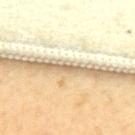<case>
  <biopsy_status>not biopsied; imaged during a skin examination</biopsy_status>
  <image>
    <source>total-body photography crop</source>
    <field_of_view_mm>15</field_of_view_mm>
  </image>
  <patient>
    <sex>female</sex>
    <age_approx>60</age_approx>
  </patient>
  <lighting>cross-polarized</lighting>
  <site>upper back</site>
  <automated_metrics>
    <area_mm2_approx>1.0</area_mm2_approx>
    <shape_asymmetry>0.55</shape_asymmetry>
  </automated_metrics>
  <lesion_size>
    <long_diameter_mm_approx>1.5</long_diameter_mm_approx>
  </lesion_size>
</case>